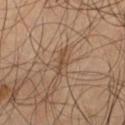No biopsy was performed on this lesion — it was imaged during a full skin examination and was not determined to be concerning. The lesion is on the left thigh. About 3 mm across. A male patient, aged around 55. Captured under cross-polarized illumination. A lesion tile, about 15 mm wide, cut from a 3D total-body photograph.